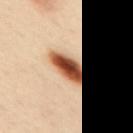biopsy status: total-body-photography surveillance lesion; no biopsy | patient: female, aged 38 to 42 | body site: the upper back | acquisition: total-body-photography crop, ~15 mm field of view.